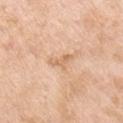This lesion was catalogued during total-body skin photography and was not selected for biopsy. This image is a 15 mm lesion crop taken from a total-body photograph. From the right upper arm. Imaged with white-light lighting. The recorded lesion diameter is about 3 mm. A male patient approximately 55 years of age.